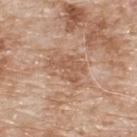Context: Approximately 4.5 mm at its widest. The subject is a male roughly 80 years of age. The tile uses white-light illumination. From the upper back. A region of skin cropped from a whole-body photographic capture, roughly 15 mm wide.